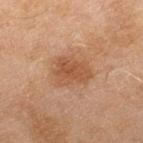* workup — imaged on a skin check; not biopsied
* anatomic site — the right lower leg
* automated lesion analysis — a lesion color around L≈48 a*≈21 b*≈32 in CIELAB, a lesion–skin lightness drop of about 8, and a normalized border contrast of about 6.5; a border-irregularity rating of about 3/10, a color-variation rating of about 2.5/10, and peripheral color asymmetry of about 1; an automated nevus-likeness rating near 45 out of 100 and lesion-presence confidence of about 100/100
* subject — female, approximately 60 years of age
* imaging modality — ~15 mm crop, total-body skin-cancer survey